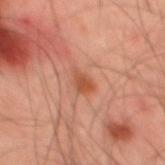follow-up: imaged on a skin check; not biopsied
automated lesion analysis: an area of roughly 3.5 mm², an eccentricity of roughly 0.7, and a shape-asymmetry score of about 0.35 (0 = symmetric); an average lesion color of about L≈49 a*≈27 b*≈33 (CIELAB) and a normalized border contrast of about 7; lesion-presence confidence of about 100/100
location: the mid back
patient: male, aged 43–47
image source: total-body-photography crop, ~15 mm field of view
lesion size: ~2.5 mm (longest diameter)